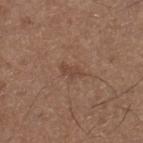<lesion>
<site>right lower leg</site>
<lighting>white-light</lighting>
<automated_metrics>
  <area_mm2_approx>3.0</area_mm2_approx>
  <eccentricity>0.85</eccentricity>
  <cielab_L>44</cielab_L>
  <cielab_a>18</cielab_a>
  <cielab_b>27</cielab_b>
  <vs_skin_darker_L>7.0</vs_skin_darker_L>
  <vs_skin_contrast_norm>5.5</vs_skin_contrast_norm>
  <border_irregularity_0_10>4.0</border_irregularity_0_10>
  <color_variation_0_10>0.5</color_variation_0_10>
  <nevus_likeness_0_100>0</nevus_likeness_0_100>
  <lesion_detection_confidence_0_100>100</lesion_detection_confidence_0_100>
</automated_metrics>
<patient>
  <sex>male</sex>
  <age_approx>75</age_approx>
</patient>
<lesion_size>
  <long_diameter_mm_approx>2.5</long_diameter_mm_approx>
</lesion_size>
<image>
  <source>total-body photography crop</source>
  <field_of_view_mm>15</field_of_view_mm>
</image>
</lesion>